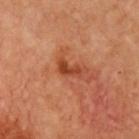biopsy_status: not biopsied; imaged during a skin examination
lesion_size:
  long_diameter_mm_approx: 3.5
image:
  source: total-body photography crop
  field_of_view_mm: 15
site: chest
patient:
  sex: female
  age_approx: 55
lighting: cross-polarized